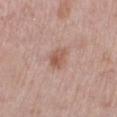{
  "biopsy_status": "not biopsied; imaged during a skin examination",
  "site": "leg",
  "automated_metrics": {
    "area_mm2_approx": 4.5,
    "eccentricity": 0.6,
    "border_irregularity_0_10": 2.0,
    "color_variation_0_10": 4.0,
    "peripheral_color_asymmetry": 1.5
  },
  "lighting": "white-light",
  "patient": {
    "sex": "female",
    "age_approx": 40
  },
  "image": {
    "source": "total-body photography crop",
    "field_of_view_mm": 15
  }
}